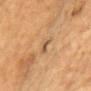Recorded during total-body skin imaging; not selected for excision or biopsy. This is a cross-polarized tile. About 3.5 mm across. Located on the chest. This image is a 15 mm lesion crop taken from a total-body photograph. A male patient approximately 85 years of age or older.